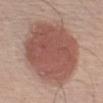Captured during whole-body skin photography for melanoma surveillance; the lesion was not biopsied.
About 10.5 mm across.
A region of skin cropped from a whole-body photographic capture, roughly 15 mm wide.
The lesion is on the left upper arm.
A male subject, in their mid-50s.
This is a white-light tile.
Automated tile analysis of the lesion measured a footprint of about 60 mm². It also reported a lesion color around L≈53 a*≈22 b*≈25 in CIELAB, about 13 CIELAB-L* units darker than the surrounding skin, and a normalized lesion–skin contrast near 8.5.